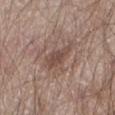patient = male, aged 78 to 82 | imaging modality = ~15 mm tile from a whole-body skin photo | size = about 5 mm | automated metrics = about 9 CIELAB-L* units darker than the surrounding skin and a normalized lesion–skin contrast near 7; a lesion-detection confidence of about 85/100 | tile lighting = white-light illumination | anatomic site = the right upper arm.